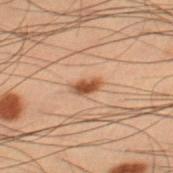Notes:
– notes — no biopsy performed (imaged during a skin exam)
– lighting — cross-polarized
– imaging modality — ~15 mm tile from a whole-body skin photo
– body site — the right thigh
– patient — male, aged approximately 55
– lesion diameter — ≈3 mm